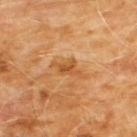Part of a total-body skin-imaging series; this lesion was reviewed on a skin check and was not flagged for biopsy. From the chest. Cropped from a total-body skin-imaging series; the visible field is about 15 mm. Longest diameter approximately 4 mm. The patient is a male about 60 years old.